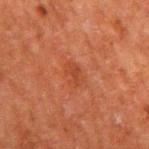Imaged during a routine full-body skin examination; the lesion was not biopsied and no histopathology is available. Captured under cross-polarized illumination. This image is a 15 mm lesion crop taken from a total-body photograph. A male patient, in their 60s. On the right upper arm.